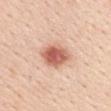Impression:
Captured during whole-body skin photography for melanoma surveillance; the lesion was not biopsied.
Clinical summary:
Captured under white-light illumination. The subject is a female aged 43–47. This image is a 15 mm lesion crop taken from a total-body photograph. Approximately 4.5 mm at its widest. The lesion is located on the mid back.